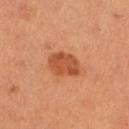subject: female, aged 23–27
automated metrics: an area of roughly 9 mm² and an outline eccentricity of about 0.75 (0 = round, 1 = elongated); a lesion–skin lightness drop of about 11 and a normalized border contrast of about 8
lesion diameter: about 4 mm
image source: ~15 mm crop, total-body skin-cancer survey
location: the left lower leg